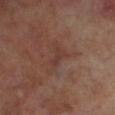{"lesion_size": {"long_diameter_mm_approx": 2.5}, "patient": {"sex": "male", "age_approx": 70}, "image": {"source": "total-body photography crop", "field_of_view_mm": 15}, "site": "left lower leg", "lighting": "cross-polarized"}